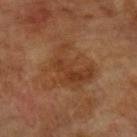Assessment:
The lesion was tiled from a total-body skin photograph and was not biopsied.
Image and clinical context:
The lesion is on the left upper arm. A 15 mm close-up extracted from a 3D total-body photography capture. The total-body-photography lesion software estimated two-axis asymmetry of about 0.45. It also reported a lesion–skin lightness drop of about 7. It also reported a lesion-detection confidence of about 100/100. The recorded lesion diameter is about 6.5 mm. A male patient, aged approximately 70.